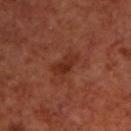Impression: The lesion was tiled from a total-body skin photograph and was not biopsied. Image and clinical context: A 15 mm crop from a total-body photograph taken for skin-cancer surveillance. The total-body-photography lesion software estimated an area of roughly 4 mm², a shape eccentricity near 0.9, and a shape-asymmetry score of about 0.35 (0 = symmetric). The analysis additionally found an automated nevus-likeness rating near 0 out of 100 and lesion-presence confidence of about 100/100. The lesion is on the upper back. This is a cross-polarized tile. About 3.5 mm across. A male patient, aged 68 to 72.